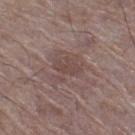follow-up: no biopsy performed (imaged during a skin exam)
image: 15 mm crop, total-body photography
anatomic site: the left lower leg
patient: male, in their mid-60s